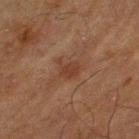Impression: Captured during whole-body skin photography for melanoma surveillance; the lesion was not biopsied. Context: Captured under cross-polarized illumination. A 15 mm close-up tile from a total-body photography series done for melanoma screening. The lesion is on the leg. Automated tile analysis of the lesion measured a color-variation rating of about 1.5/10 and a peripheral color-asymmetry measure near 0.5. A male patient approximately 65 years of age.